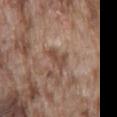Assessment:
The lesion was tiled from a total-body skin photograph and was not biopsied.
Image and clinical context:
The subject is a male in their mid-70s. This is a white-light tile. On the back. A 15 mm close-up tile from a total-body photography series done for melanoma screening.